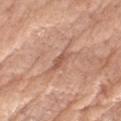biopsy status: imaged on a skin check; not biopsied | imaging modality: ~15 mm tile from a whole-body skin photo | subject: female, aged around 75 | automated lesion analysis: a footprint of about 2.5 mm² and a shape-asymmetry score of about 0.2 (0 = symmetric); a lesion color around L≈55 a*≈23 b*≈30 in CIELAB, roughly 9 lightness units darker than nearby skin, and a normalized border contrast of about 6 | site: the right thigh | lesion diameter: ~2.5 mm (longest diameter).